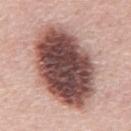notes=catalogued during a skin exam; not biopsied | imaging modality=total-body-photography crop, ~15 mm field of view | patient=male, in their mid-60s | diameter=~11 mm (longest diameter) | image-analysis metrics=a lesion color around L≈49 a*≈21 b*≈22 in CIELAB, about 23 CIELAB-L* units darker than the surrounding skin, and a normalized lesion–skin contrast near 14.5; border irregularity of about 1.5 on a 0–10 scale, a color-variation rating of about 9.5/10, and radial color variation of about 3; an automated nevus-likeness rating near 50 out of 100 | body site=the mid back.